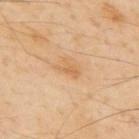  biopsy_status: not biopsied; imaged during a skin examination
  lesion_size:
    long_diameter_mm_approx: 2.5
  patient:
    sex: male
    age_approx: 55
  lighting: cross-polarized
  site: back
  image:
    source: total-body photography crop
    field_of_view_mm: 15